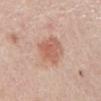Recorded during total-body skin imaging; not selected for excision or biopsy. A female patient roughly 65 years of age. A 15 mm close-up tile from a total-body photography series done for melanoma screening. The lesion's longest dimension is about 4.5 mm. Captured under white-light illumination. From the chest.